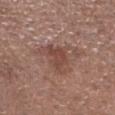Q: Is there a histopathology result?
A: imaged on a skin check; not biopsied
Q: What did automated image analysis measure?
A: a footprint of about 7.5 mm² and an eccentricity of roughly 0.75; a lesion color around L≈45 a*≈21 b*≈25 in CIELAB, about 8 CIELAB-L* units darker than the surrounding skin, and a lesion-to-skin contrast of about 6.5 (normalized; higher = more distinct)
Q: How large is the lesion?
A: ≈4 mm
Q: How was this image acquired?
A: 15 mm crop, total-body photography
Q: Patient demographics?
A: male, in their mid- to late 30s
Q: Where on the body is the lesion?
A: the head or neck
Q: Illumination type?
A: white-light